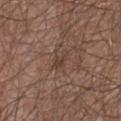Q: Is there a histopathology result?
A: imaged on a skin check; not biopsied
Q: Lesion location?
A: the chest
Q: Who is the patient?
A: male, aged 63–67
Q: How was this image acquired?
A: 15 mm crop, total-body photography
Q: Illumination type?
A: white-light
Q: Automated lesion metrics?
A: an eccentricity of roughly 0.9 and a symmetry-axis asymmetry near 0.4; a lesion color around L≈40 a*≈16 b*≈24 in CIELAB and roughly 7 lightness units darker than nearby skin